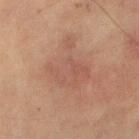Assessment:
The lesion was tiled from a total-body skin photograph and was not biopsied.
Context:
A male subject, aged approximately 75. Measured at roughly 6 mm in maximum diameter. A roughly 15 mm field-of-view crop from a total-body skin photograph. Captured under cross-polarized illumination. The lesion is on the right upper arm. The total-body-photography lesion software estimated a border-irregularity rating of about 7/10 and internal color variation of about 3 on a 0–10 scale. The software also gave a classifier nevus-likeness of about 0/100 and a lesion-detection confidence of about 100/100.